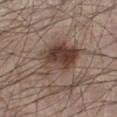Notes:
* workup: total-body-photography surveillance lesion; no biopsy
* lesion size: ~7.5 mm (longest diameter)
* anatomic site: the left lower leg
* subject: male, approximately 60 years of age
* image source: total-body-photography crop, ~15 mm field of view
* tile lighting: white-light